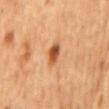{"biopsy_status": "not biopsied; imaged during a skin examination", "patient": {"sex": "male", "age_approx": 65}, "image": {"source": "total-body photography crop", "field_of_view_mm": 15}, "site": "mid back"}